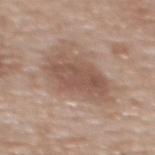Case summary:
* site: the upper back
* image-analysis metrics: an average lesion color of about L≈53 a*≈18 b*≈26 (CIELAB), a lesion–skin lightness drop of about 10, and a lesion-to-skin contrast of about 7 (normalized; higher = more distinct); a color-variation rating of about 3.5/10 and a peripheral color-asymmetry measure near 1; lesion-presence confidence of about 100/100
* acquisition: ~15 mm crop, total-body skin-cancer survey
* lesion size: ≈6.5 mm
* lighting: white-light
* patient: female, aged around 60